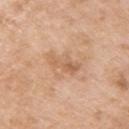Impression:
The lesion was photographed on a routine skin check and not biopsied; there is no pathology result.
Image and clinical context:
This image is a 15 mm lesion crop taken from a total-body photograph. The patient is a female in their mid-70s. Imaged with white-light lighting. The lesion is located on the right upper arm. The total-body-photography lesion software estimated an area of roughly 5.5 mm², an outline eccentricity of about 0.95 (0 = round, 1 = elongated), and a symmetry-axis asymmetry near 0.3. The software also gave a lesion color around L≈61 a*≈20 b*≈34 in CIELAB, about 8 CIELAB-L* units darker than the surrounding skin, and a normalized lesion–skin contrast near 5.5.